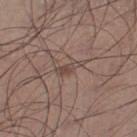The lesion was tiled from a total-body skin photograph and was not biopsied. A roughly 15 mm field-of-view crop from a total-body skin photograph. Located on the right lower leg. The subject is a male about 45 years old. Captured under white-light illumination. Automated image analysis of the tile measured a border-irregularity rating of about 4/10 and a within-lesion color-variation index near 1.5/10.